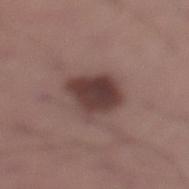{
  "biopsy_status": "not biopsied; imaged during a skin examination",
  "site": "leg",
  "patient": {
    "sex": "male",
    "age_approx": 35
  },
  "lighting": "white-light",
  "image": {
    "source": "total-body photography crop",
    "field_of_view_mm": 15
  },
  "lesion_size": {
    "long_diameter_mm_approx": 5.0
  }
}